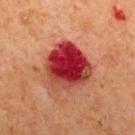The lesion was photographed on a routine skin check and not biopsied; there is no pathology result. Automated tile analysis of the lesion measured a lesion color around L≈34 a*≈37 b*≈27 in CIELAB and a normalized border contrast of about 14. The software also gave an automated nevus-likeness rating near 0 out of 100 and lesion-presence confidence of about 100/100. The patient is a male aged 63 to 67. A roughly 15 mm field-of-view crop from a total-body skin photograph. The lesion is located on the mid back. Approximately 5.5 mm at its widest.